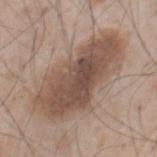biopsy status = imaged on a skin check; not biopsied | diameter = ≈10.5 mm | patient = male, aged 58–62 | site = the chest | acquisition = ~15 mm tile from a whole-body skin photo.